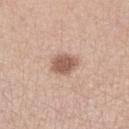Imaged during a routine full-body skin examination; the lesion was not biopsied and no histopathology is available. A roughly 15 mm field-of-view crop from a total-body skin photograph. A female subject aged approximately 60. The lesion is on the left lower leg.